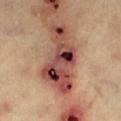<case>
  <biopsy_status>not biopsied; imaged during a skin examination</biopsy_status>
  <image>
    <source>total-body photography crop</source>
    <field_of_view_mm>15</field_of_view_mm>
  </image>
  <patient>
    <age_approx>60</age_approx>
  </patient>
  <site>left lower leg</site>
</case>